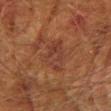| key | value |
|---|---|
| workup | no biopsy performed (imaged during a skin exam) |
| diameter | about 5.5 mm |
| image source | ~15 mm tile from a whole-body skin photo |
| automated lesion analysis | an average lesion color of about L≈31 a*≈21 b*≈25 (CIELAB) and about 5 CIELAB-L* units darker than the surrounding skin; a color-variation rating of about 4/10 |
| lighting | cross-polarized |
| body site | the right upper arm |
| subject | male, aged 73 to 77 |